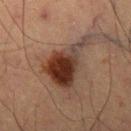notes=imaged on a skin check; not biopsied
anatomic site=the leg
image=~15 mm tile from a whole-body skin photo
subject=male, aged approximately 65